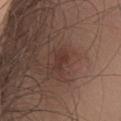Automated tile analysis of the lesion measured a footprint of about 3.5 mm², an outline eccentricity of about 0.85 (0 = round, 1 = elongated), and a symmetry-axis asymmetry near 0.3. And it measured an average lesion color of about L≈34 a*≈19 b*≈23 (CIELAB), about 6 CIELAB-L* units darker than the surrounding skin, and a normalized border contrast of about 6. A lesion tile, about 15 mm wide, cut from a 3D total-body photograph. The subject is a male roughly 55 years of age. Measured at roughly 2.5 mm in maximum diameter. This is a white-light tile. Located on the chest.